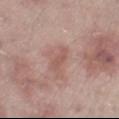  biopsy_status: not biopsied; imaged during a skin examination
  lighting: white-light
  image:
    source: total-body photography crop
    field_of_view_mm: 15
  patient:
    sex: male
    age_approx: 65
  automated_metrics:
    area_mm2_approx: 3.5
    eccentricity: 0.9
    shape_asymmetry: 0.4
    vs_skin_darker_L: 7.0
  site: right thigh